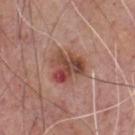{
  "biopsy_status": "not biopsied; imaged during a skin examination",
  "automated_metrics": {
    "nevus_likeness_0_100": 15,
    "lesion_detection_confidence_0_100": 100
  },
  "lesion_size": {
    "long_diameter_mm_approx": 4.5
  },
  "lighting": "white-light",
  "site": "front of the torso",
  "patient": {
    "sex": "male",
    "age_approx": 75
  },
  "image": {
    "source": "total-body photography crop",
    "field_of_view_mm": 15
  }
}